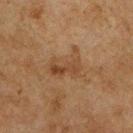<tbp_lesion>
<biopsy_status>not biopsied; imaged during a skin examination</biopsy_status>
<site>chest</site>
<automated_metrics>
  <area_mm2_approx>12.0</area_mm2_approx>
  <eccentricity>0.75</eccentricity>
  <shape_asymmetry>0.35</shape_asymmetry>
  <peripheral_color_asymmetry>2.0</peripheral_color_asymmetry>
  <nevus_likeness_0_100>0</nevus_likeness_0_100>
  <lesion_detection_confidence_0_100>100</lesion_detection_confidence_0_100>
</automated_metrics>
<image>
  <source>total-body photography crop</source>
  <field_of_view_mm>15</field_of_view_mm>
</image>
<patient>
  <sex>male</sex>
  <age_approx>75</age_approx>
</patient>
<lighting>cross-polarized</lighting>
<lesion_size>
  <long_diameter_mm_approx>4.5</long_diameter_mm_approx>
</lesion_size>
</tbp_lesion>